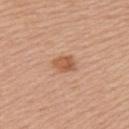biopsy status — catalogued during a skin exam; not biopsied | subject — female, aged 53 to 57 | image source — ~15 mm tile from a whole-body skin photo | location — the arm | lighting — white-light illumination | image-analysis metrics — a lesion color around L≈57 a*≈24 b*≈35 in CIELAB, a lesion–skin lightness drop of about 10, and a normalized border contrast of about 7.5; a border-irregularity rating of about 2/10 and internal color variation of about 3.5 on a 0–10 scale; a detector confidence of about 100 out of 100 that the crop contains a lesion.